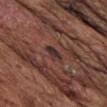No biopsy was performed on this lesion — it was imaged during a full skin examination and was not determined to be concerning.
Measured at roughly 2.5 mm in maximum diameter.
The patient is a male approximately 75 years of age.
The tile uses white-light illumination.
Cropped from a total-body skin-imaging series; the visible field is about 15 mm.
On the chest.
Automated tile analysis of the lesion measured an average lesion color of about L≈31 a*≈17 b*≈18 (CIELAB), a lesion–skin lightness drop of about 9, and a normalized border contrast of about 10. It also reported a border-irregularity rating of about 3.5/10 and a peripheral color-asymmetry measure near 0.5.